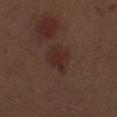Part of a total-body skin-imaging series; this lesion was reviewed on a skin check and was not flagged for biopsy.
A 15 mm crop from a total-body photograph taken for skin-cancer surveillance.
About 4 mm across.
Automated tile analysis of the lesion measured an area of roughly 8.5 mm², an eccentricity of roughly 0.75, and a symmetry-axis asymmetry near 0.2. The analysis additionally found a lesion–skin lightness drop of about 6 and a normalized border contrast of about 6.5. The analysis additionally found a border-irregularity index near 2.5/10, internal color variation of about 3 on a 0–10 scale, and peripheral color asymmetry of about 1.
A male subject, aged 68–72.
The lesion is located on the left thigh.